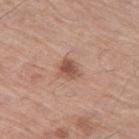| field | value |
|---|---|
| notes | imaged on a skin check; not biopsied |
| imaging modality | ~15 mm tile from a whole-body skin photo |
| lesion size | ≈2.5 mm |
| body site | the left thigh |
| tile lighting | white-light |
| subject | male, roughly 70 years of age |